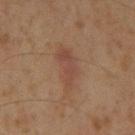Image and clinical context: Captured under cross-polarized illumination. A male subject, aged 58–62. About 6 mm across. The lesion is on the mid back. The lesion-visualizer software estimated roughly 6 lightness units darker than nearby skin and a lesion-to-skin contrast of about 5 (normalized; higher = more distinct). And it measured a border-irregularity rating of about 4/10, a color-variation rating of about 2.5/10, and a peripheral color-asymmetry measure near 1. And it measured a nevus-likeness score of about 0/100. A 15 mm close-up extracted from a 3D total-body photography capture.